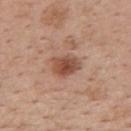- workup · no biopsy performed (imaged during a skin exam)
- patient · female, roughly 50 years of age
- site · the upper back
- imaging modality · ~15 mm crop, total-body skin-cancer survey